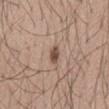No biopsy was performed on this lesion — it was imaged during a full skin examination and was not determined to be concerning. Approximately 3 mm at its widest. This is a white-light tile. Automated tile analysis of the lesion measured an average lesion color of about L≈49 a*≈18 b*≈25 (CIELAB), a lesion–skin lightness drop of about 14, and a normalized lesion–skin contrast near 9.5. The software also gave a border-irregularity index near 3.5/10, a color-variation rating of about 0/10, and radial color variation of about 0. And it measured an automated nevus-likeness rating near 95 out of 100 and a detector confidence of about 100 out of 100 that the crop contains a lesion. A 15 mm close-up extracted from a 3D total-body photography capture. The patient is a male aged around 40. Located on the mid back.